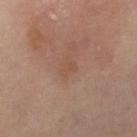No biopsy was performed on this lesion — it was imaged during a full skin examination and was not determined to be concerning. On the right lower leg. A female subject, approximately 40 years of age. This is a cross-polarized tile. This image is a 15 mm lesion crop taken from a total-body photograph.